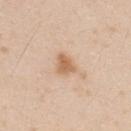Clinical impression: Captured during whole-body skin photography for melanoma surveillance; the lesion was not biopsied. Clinical summary: A male patient, aged 23 to 27. The lesion is located on the upper back. A roughly 15 mm field-of-view crop from a total-body skin photograph. An algorithmic analysis of the crop reported a mean CIELAB color near L≈63 a*≈19 b*≈35 and about 11 CIELAB-L* units darker than the surrounding skin. The software also gave a border-irregularity index near 3/10 and a within-lesion color-variation index near 2/10. The analysis additionally found a nevus-likeness score of about 80/100 and a lesion-detection confidence of about 100/100. This is a white-light tile. Measured at roughly 2.5 mm in maximum diameter.